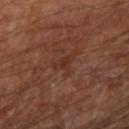The lesion was tiled from a total-body skin photograph and was not biopsied. Approximately 3 mm at its widest. Located on the left arm. Cropped from a whole-body photographic skin survey; the tile spans about 15 mm. The subject is a male approximately 65 years of age.